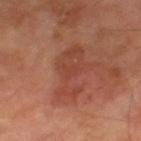Part of a total-body skin-imaging series; this lesion was reviewed on a skin check and was not flagged for biopsy.